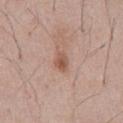notes = total-body-photography surveillance lesion; no biopsy
size = about 2.5 mm
subject = male, aged 53 to 57
TBP lesion metrics = roughly 10 lightness units darker than nearby skin and a normalized border contrast of about 7; a within-lesion color-variation index near 4/10; a nevus-likeness score of about 65/100 and a detector confidence of about 100 out of 100 that the crop contains a lesion
location = the abdomen
acquisition = total-body-photography crop, ~15 mm field of view
lighting = white-light illumination